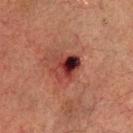imaging modality=total-body-photography crop, ~15 mm field of view
lesion size=~3.5 mm (longest diameter)
body site=the head or neck
automated lesion analysis=a within-lesion color-variation index near 10/10 and radial color variation of about 7
patient=male, roughly 65 years of age
illumination=cross-polarized illumination
biopsy diagnosis=a nodular basal cell carcinoma (malignant)